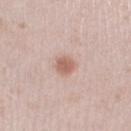notes: imaged on a skin check; not biopsied
subject: female, in their mid- to late 20s
automated lesion analysis: an area of roughly 4 mm², an eccentricity of roughly 0.6, and a shape-asymmetry score of about 0.25 (0 = symmetric)
diameter: ≈2.5 mm
image source: ~15 mm tile from a whole-body skin photo
anatomic site: the leg
illumination: white-light